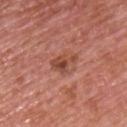Part of a total-body skin-imaging series; this lesion was reviewed on a skin check and was not flagged for biopsy.
Located on the front of the torso.
A male subject aged approximately 70.
A 15 mm close-up extracted from a 3D total-body photography capture.
Captured under white-light illumination.